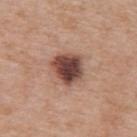Imaged during a routine full-body skin examination; the lesion was not biopsied and no histopathology is available. The total-body-photography lesion software estimated a mean CIELAB color near L≈44 a*≈21 b*≈24 and a normalized lesion–skin contrast near 13. Located on the upper back. The lesion's longest dimension is about 4 mm. A region of skin cropped from a whole-body photographic capture, roughly 15 mm wide. The subject is a female about 40 years old.